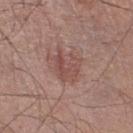Assessment: Imaged during a routine full-body skin examination; the lesion was not biopsied and no histopathology is available. Context: This is a white-light tile. Approximately 4 mm at its widest. From the right lower leg. An algorithmic analysis of the crop reported about 7 CIELAB-L* units darker than the surrounding skin. A roughly 15 mm field-of-view crop from a total-body skin photograph. A male subject aged approximately 55.